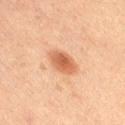Imaged during a routine full-body skin examination; the lesion was not biopsied and no histopathology is available. Located on the right thigh. The tile uses cross-polarized illumination. A female subject, roughly 20 years of age. A lesion tile, about 15 mm wide, cut from a 3D total-body photograph.